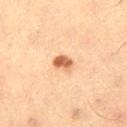The lesion was photographed on a routine skin check and not biopsied; there is no pathology result.
This image is a 15 mm lesion crop taken from a total-body photograph.
The patient is a male aged 58–62.
Longest diameter approximately 2.5 mm.
The lesion is on the right thigh.